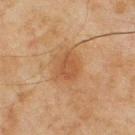Impression: This lesion was catalogued during total-body skin photography and was not selected for biopsy. Context: A 15 mm close-up tile from a total-body photography series done for melanoma screening. On the upper back. A male subject, aged 43–47. The tile uses cross-polarized illumination. An algorithmic analysis of the crop reported a lesion area of about 6.5 mm², a shape eccentricity near 0.5, and a shape-asymmetry score of about 0.3 (0 = symmetric). It also reported a mean CIELAB color near L≈42 a*≈19 b*≈31, roughly 7 lightness units darker than nearby skin, and a normalized lesion–skin contrast near 5.5. The software also gave a border-irregularity index near 2.5/10, a color-variation rating of about 2/10, and radial color variation of about 0.5. It also reported a classifier nevus-likeness of about 45/100 and a detector confidence of about 100 out of 100 that the crop contains a lesion. The lesion's longest dimension is about 3 mm.